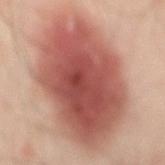• biopsy status — imaged on a skin check; not biopsied